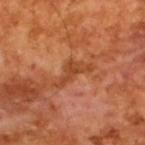Captured during whole-body skin photography for melanoma surveillance; the lesion was not biopsied. A close-up tile cropped from a whole-body skin photograph, about 15 mm across. A male patient, aged 63 to 67. Measured at roughly 5 mm in maximum diameter. This is a cross-polarized tile.